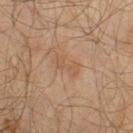Findings:
- notes — total-body-photography surveillance lesion; no biopsy
- location — the arm
- image — ~15 mm tile from a whole-body skin photo
- subject — male, aged 63 to 67